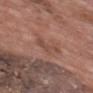Captured during whole-body skin photography for melanoma surveillance; the lesion was not biopsied. A male subject, aged 68 to 72. This image is a 15 mm lesion crop taken from a total-body photograph. The lesion's longest dimension is about 3 mm. Imaged with white-light lighting. On the back.